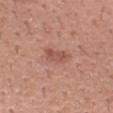Assessment:
Part of a total-body skin-imaging series; this lesion was reviewed on a skin check and was not flagged for biopsy.
Context:
Cropped from a total-body skin-imaging series; the visible field is about 15 mm. The patient is a male roughly 40 years of age. From the right forearm.